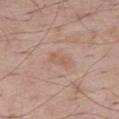The lesion was tiled from a total-body skin photograph and was not biopsied.